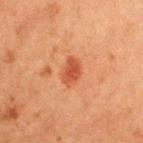| field | value |
|---|---|
| notes | total-body-photography surveillance lesion; no biopsy |
| patient | female, aged approximately 50 |
| lesion diameter | ≈3 mm |
| tile lighting | cross-polarized |
| body site | the left upper arm |
| image | ~15 mm tile from a whole-body skin photo |